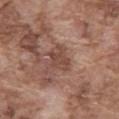Imaged during a routine full-body skin examination; the lesion was not biopsied and no histopathology is available. Cropped from a whole-body photographic skin survey; the tile spans about 15 mm. A male patient, in their mid- to late 70s. Located on the abdomen.